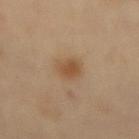<lesion>
  <lesion_size>
    <long_diameter_mm_approx>3.0</long_diameter_mm_approx>
  </lesion_size>
  <patient>
    <sex>female</sex>
    <age_approx>60</age_approx>
  </patient>
  <site>back</site>
  <image>
    <source>total-body photography crop</source>
    <field_of_view_mm>15</field_of_view_mm>
  </image>
  <lighting>cross-polarized</lighting>
  <automated_metrics>
    <area_mm2_approx>5.0</area_mm2_approx>
    <eccentricity>0.75</eccentricity>
    <shape_asymmetry>0.2</shape_asymmetry>
    <cielab_L>51</cielab_L>
    <cielab_a>18</cielab_a>
    <cielab_b>35</cielab_b>
    <vs_skin_darker_L>9.0</vs_skin_darker_L>
    <vs_skin_contrast_norm>7.5</vs_skin_contrast_norm>
  </automated_metrics>
</lesion>